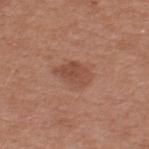notes: catalogued during a skin exam; not biopsied
image source: ~15 mm tile from a whole-body skin photo
illumination: white-light
subject: male, aged around 55
site: the upper back